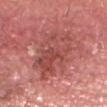Clinical impression:
This lesion was catalogued during total-body skin photography and was not selected for biopsy.
Background:
A 15 mm close-up tile from a total-body photography series done for melanoma screening. This is a white-light tile. A male patient, aged 63–67. The lesion is on the head or neck.